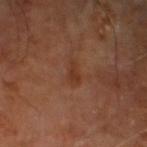Part of a total-body skin-imaging series; this lesion was reviewed on a skin check and was not flagged for biopsy. The lesion's longest dimension is about 2.5 mm. Imaged with cross-polarized lighting. The lesion is on the right leg. A male subject, aged 58–62. The lesion-visualizer software estimated a mean CIELAB color near L≈33 a*≈22 b*≈28 and a lesion-to-skin contrast of about 6 (normalized; higher = more distinct). And it measured a border-irregularity index near 3/10, a within-lesion color-variation index near 0.5/10, and a peripheral color-asymmetry measure near 0. It also reported a classifier nevus-likeness of about 0/100. A 15 mm crop from a total-body photograph taken for skin-cancer surveillance.